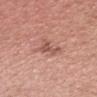Recorded during total-body skin imaging; not selected for excision or biopsy.
Imaged with white-light lighting.
A 15 mm close-up tile from a total-body photography series done for melanoma screening.
The patient is a male aged around 30.
On the head or neck.
Longest diameter approximately 2.5 mm.